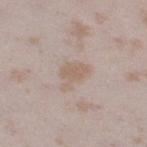Clinical impression:
Recorded during total-body skin imaging; not selected for excision or biopsy.
Background:
The lesion is on the right thigh. A lesion tile, about 15 mm wide, cut from a 3D total-body photograph. The subject is a female in their mid- to late 20s. The lesion-visualizer software estimated an area of roughly 5.5 mm² and two-axis asymmetry of about 0.35. The software also gave a lesion color around L≈60 a*≈14 b*≈25 in CIELAB, a lesion–skin lightness drop of about 7, and a lesion-to-skin contrast of about 5.5 (normalized; higher = more distinct). And it measured a peripheral color-asymmetry measure near 0.5. The analysis additionally found a detector confidence of about 100 out of 100 that the crop contains a lesion. The recorded lesion diameter is about 3.5 mm. The tile uses white-light illumination.